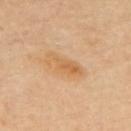Q: Was this lesion biopsied?
A: no biopsy performed (imaged during a skin exam)
Q: What kind of image is this?
A: total-body-photography crop, ~15 mm field of view
Q: Lesion location?
A: the upper back
Q: Illumination type?
A: cross-polarized illumination
Q: Patient demographics?
A: female, aged 58–62
Q: Lesion size?
A: ~5 mm (longest diameter)